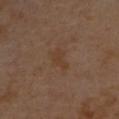Assessment: The lesion was tiled from a total-body skin photograph and was not biopsied. Acquisition and patient details: Captured under cross-polarized illumination. A lesion tile, about 15 mm wide, cut from a 3D total-body photograph. A male subject, roughly 55 years of age. The lesion is on the upper back. The lesion's longest dimension is about 3 mm.